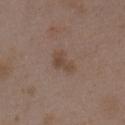Findings:
• image source: total-body-photography crop, ~15 mm field of view
• TBP lesion metrics: a footprint of about 4.5 mm² and an outline eccentricity of about 0.85 (0 = round, 1 = elongated); an average lesion color of about L≈45 a*≈16 b*≈25 (CIELAB), a lesion–skin lightness drop of about 7, and a lesion-to-skin contrast of about 6.5 (normalized; higher = more distinct); a border-irregularity index near 4.5/10, a within-lesion color-variation index near 1.5/10, and radial color variation of about 0.5; a nevus-likeness score of about 5/100 and lesion-presence confidence of about 100/100
• patient: female, about 35 years old
• illumination: white-light illumination
• site: the chest